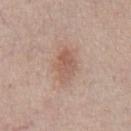automated metrics = an area of roughly 7 mm², a shape eccentricity near 0.75, and two-axis asymmetry of about 0.2; about 9 CIELAB-L* units darker than the surrounding skin and a normalized border contrast of about 6; a border-irregularity rating of about 2/10 and a peripheral color-asymmetry measure near 1; a nevus-likeness score of about 45/100 and a lesion-detection confidence of about 100/100 | anatomic site = the chest | diameter = about 4 mm | illumination = white-light illumination | acquisition = ~15 mm crop, total-body skin-cancer survey | subject = male, approximately 55 years of age.